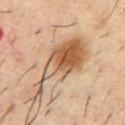biopsy status: no biopsy performed (imaged during a skin exam) | subject: male, aged 33–37 | image: ~15 mm tile from a whole-body skin photo | site: the chest.